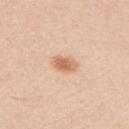anatomic site: the upper back | lighting: white-light illumination | patient: male, in their mid-40s | imaging modality: ~15 mm tile from a whole-body skin photo | image-analysis metrics: two-axis asymmetry of about 0.2; a lesion color around L≈66 a*≈21 b*≈35 in CIELAB, roughly 12 lightness units darker than nearby skin, and a lesion-to-skin contrast of about 7.5 (normalized; higher = more distinct); a border-irregularity index near 1.5/10 and internal color variation of about 3 on a 0–10 scale; a lesion-detection confidence of about 100/100.